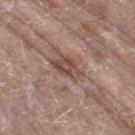Notes:
* biopsy status: imaged on a skin check; not biopsied
* diameter: ≈3.5 mm
* image source: total-body-photography crop, ~15 mm field of view
* subject: male, approximately 80 years of age
* body site: the right thigh
* automated lesion analysis: a lesion area of about 6 mm² and a symmetry-axis asymmetry near 0.35